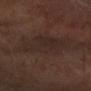workup — imaged on a skin check; not biopsied
lesion diameter — ≈6.5 mm
imaging modality — ~15 mm crop, total-body skin-cancer survey
subject — male, approximately 55 years of age
body site — the right forearm
lighting — cross-polarized
image-analysis metrics — an area of roughly 19 mm², an outline eccentricity of about 0.8 (0 = round, 1 = elongated), and a shape-asymmetry score of about 0.3 (0 = symmetric); a mean CIELAB color near L≈24 a*≈13 b*≈18, roughly 4 lightness units darker than nearby skin, and a normalized border contrast of about 5; a border-irregularity index near 4/10, a color-variation rating of about 2.5/10, and peripheral color asymmetry of about 0.5